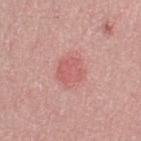This lesion was catalogued during total-body skin photography and was not selected for biopsy. Longest diameter approximately 3 mm. Cropped from a whole-body photographic skin survey; the tile spans about 15 mm. A female patient roughly 65 years of age. From the right thigh.